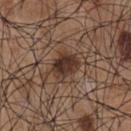The lesion was tiled from a total-body skin photograph and was not biopsied. Automated image analysis of the tile measured a lesion area of about 8 mm², an eccentricity of roughly 0.7, and a shape-asymmetry score of about 0.2 (0 = symmetric). It also reported an average lesion color of about L≈34 a*≈17 b*≈24 (CIELAB), roughly 12 lightness units darker than nearby skin, and a normalized border contrast of about 10.5. And it measured a border-irregularity index near 2/10, internal color variation of about 4 on a 0–10 scale, and peripheral color asymmetry of about 1.5. The lesion is on the chest. The patient is a male about 55 years old. A roughly 15 mm field-of-view crop from a total-body skin photograph.